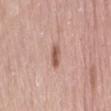- imaging modality · total-body-photography crop, ~15 mm field of view
- site · the right thigh
- illumination · white-light
- image-analysis metrics · an eccentricity of roughly 0.85 and two-axis asymmetry of about 0.3; about 12 CIELAB-L* units darker than the surrounding skin and a lesion-to-skin contrast of about 8 (normalized; higher = more distinct); lesion-presence confidence of about 100/100
- subject · female, roughly 65 years of age
- lesion diameter · ≈3 mm